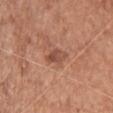Recorded during total-body skin imaging; not selected for excision or biopsy. This is a white-light tile. The subject is a female aged 38 to 42. The lesion-visualizer software estimated an area of roughly 4 mm², a shape eccentricity near 0.8, and two-axis asymmetry of about 0.3. The software also gave a within-lesion color-variation index near 4/10 and radial color variation of about 1.5. A 15 mm crop from a total-body photograph taken for skin-cancer surveillance. The lesion is on the upper back. Longest diameter approximately 3 mm.